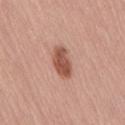Findings:
• workup — imaged on a skin check; not biopsied
• patient — female, about 45 years old
• size — about 4 mm
• image — ~15 mm crop, total-body skin-cancer survey
• anatomic site — the lower back